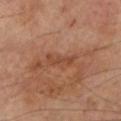Q: Is there a histopathology result?
A: imaged on a skin check; not biopsied
Q: How was this image acquired?
A: ~15 mm crop, total-body skin-cancer survey
Q: Lesion location?
A: the left lower leg
Q: What are the patient's age and sex?
A: male, aged 68–72
Q: How large is the lesion?
A: ≈3.5 mm
Q: What did automated image analysis measure?
A: an area of roughly 5 mm² and an eccentricity of roughly 0.9; a border-irregularity rating of about 4.5/10, a color-variation rating of about 1.5/10, and a peripheral color-asymmetry measure near 0.5; an automated nevus-likeness rating near 0 out of 100 and a lesion-detection confidence of about 90/100